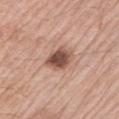subject=male, aged 73 to 77; imaging modality=~15 mm tile from a whole-body skin photo; body site=the arm; lighting=white-light illumination.